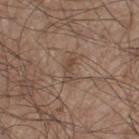Impression:
The lesion was tiled from a total-body skin photograph and was not biopsied.
Image and clinical context:
On the right thigh. The subject is a male aged around 75. Automated tile analysis of the lesion measured an area of roughly 3.5 mm², an outline eccentricity of about 0.9 (0 = round, 1 = elongated), and a shape-asymmetry score of about 0.3 (0 = symmetric). The software also gave a lesion color around L≈45 a*≈15 b*≈26 in CIELAB, about 7 CIELAB-L* units darker than the surrounding skin, and a normalized border contrast of about 6. It also reported border irregularity of about 4 on a 0–10 scale, internal color variation of about 0.5 on a 0–10 scale, and a peripheral color-asymmetry measure near 0. A 15 mm close-up tile from a total-body photography series done for melanoma screening.